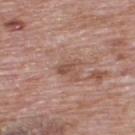biopsy_status: not biopsied; imaged during a skin examination
lesion_size:
  long_diameter_mm_approx: 2.5
site: upper back
image:
  source: total-body photography crop
  field_of_view_mm: 15
automated_metrics:
  eccentricity: 0.85
  cielab_L: 51
  cielab_a: 20
  cielab_b: 26
  vs_skin_darker_L: 9.0
  vs_skin_contrast_norm: 6.5
  border_irregularity_0_10: 2.5
  color_variation_0_10: 3.0
  nevus_likeness_0_100: 0
patient:
  sex: male
  age_approx: 70